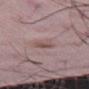Clinical impression: Captured during whole-body skin photography for melanoma surveillance; the lesion was not biopsied. Context: A roughly 15 mm field-of-view crop from a total-body skin photograph. The subject is a male aged around 50. The lesion is located on the right thigh.